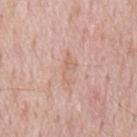{"biopsy_status": "not biopsied; imaged during a skin examination", "lesion_size": {"long_diameter_mm_approx": 3.5}, "site": "mid back", "automated_metrics": {"eccentricity": 0.9, "shape_asymmetry": 0.55, "vs_skin_darker_L": 6.0}, "lighting": "white-light", "image": {"source": "total-body photography crop", "field_of_view_mm": 15}, "patient": {"sex": "male", "age_approx": 65}}